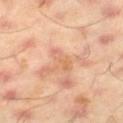  image:
    source: total-body photography crop
    field_of_view_mm: 15
  patient:
    sex: male
    age_approx: 45
  site: right thigh